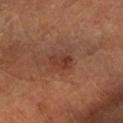The recorded lesion diameter is about 2.5 mm.
The lesion is on the right forearm.
The lesion-visualizer software estimated a footprint of about 4 mm² and a shape-asymmetry score of about 0.4 (0 = symmetric). And it measured about 7 CIELAB-L* units darker than the surrounding skin and a normalized lesion–skin contrast near 6.5.
Cropped from a whole-body photographic skin survey; the tile spans about 15 mm.
A male patient, aged 58 to 62.
Captured under cross-polarized illumination.